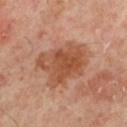Recorded during total-body skin imaging; not selected for excision or biopsy.
Imaged with cross-polarized lighting.
Measured at roughly 7 mm in maximum diameter.
The lesion is on the leg.
This image is a 15 mm lesion crop taken from a total-body photograph.
The patient is a male in their 50s.
Automated image analysis of the tile measured an area of roughly 25 mm² and a shape-asymmetry score of about 0.3 (0 = symmetric). The analysis additionally found border irregularity of about 4 on a 0–10 scale, internal color variation of about 4.5 on a 0–10 scale, and peripheral color asymmetry of about 1.5. And it measured a classifier nevus-likeness of about 5/100 and a detector confidence of about 100 out of 100 that the crop contains a lesion.